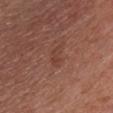Imaged during a routine full-body skin examination; the lesion was not biopsied and no histopathology is available.
Measured at roughly 3 mm in maximum diameter.
The subject is a male in their mid-50s.
Captured under white-light illumination.
From the front of the torso.
A 15 mm close-up extracted from a 3D total-body photography capture.
Automated tile analysis of the lesion measured a mean CIELAB color near L≈41 a*≈23 b*≈27, about 5 CIELAB-L* units darker than the surrounding skin, and a lesion-to-skin contrast of about 4.5 (normalized; higher = more distinct). It also reported a border-irregularity rating of about 4/10 and radial color variation of about 0.